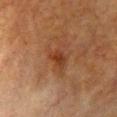Imaged during a routine full-body skin examination; the lesion was not biopsied and no histopathology is available. The lesion is on the chest. A 15 mm close-up extracted from a 3D total-body photography capture. A female subject, aged 53 to 57.